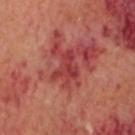Clinical impression: The lesion was photographed on a routine skin check and not biopsied; there is no pathology result. Acquisition and patient details: The lesion is on the head or neck. An algorithmic analysis of the crop reported an average lesion color of about L≈40 a*≈35 b*≈26 (CIELAB) and a normalized lesion–skin contrast near 6.5. The software also gave radial color variation of about 0.5. Captured under cross-polarized illumination. A roughly 15 mm field-of-view crop from a total-body skin photograph. About 3.5 mm across. A male patient aged around 60.